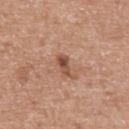Background: A 15 mm crop from a total-body photograph taken for skin-cancer surveillance. The lesion is on the upper back. A male subject, aged 53 to 57.